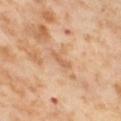This lesion was catalogued during total-body skin photography and was not selected for biopsy.
On the left thigh.
A female subject aged around 55.
About 2.5 mm across.
A 15 mm close-up extracted from a 3D total-body photography capture.
This is a cross-polarized tile.
The lesion-visualizer software estimated a lesion area of about 2.5 mm², an eccentricity of roughly 0.95, and two-axis asymmetry of about 0.4. And it measured about 8 CIELAB-L* units darker than the surrounding skin and a normalized lesion–skin contrast near 5.5. It also reported a nevus-likeness score of about 0/100 and a lesion-detection confidence of about 100/100.